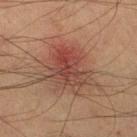Impression: Recorded during total-body skin imaging; not selected for excision or biopsy. Acquisition and patient details: Automated image analysis of the tile measured a lesion color around L≈40 a*≈21 b*≈25 in CIELAB, roughly 8 lightness units darker than nearby skin, and a normalized lesion–skin contrast near 7. The analysis additionally found an automated nevus-likeness rating near 55 out of 100 and a detector confidence of about 100 out of 100 that the crop contains a lesion. On the left lower leg. A 15 mm close-up extracted from a 3D total-body photography capture. The lesion's longest dimension is about 5.5 mm. The subject is a male aged approximately 60.